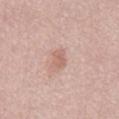biopsy_status: not biopsied; imaged during a skin examination
site: mid back
lesion_size:
  long_diameter_mm_approx: 2.5
automated_metrics:
  vs_skin_darker_L: 9.0
lighting: white-light
image:
  source: total-body photography crop
  field_of_view_mm: 15
patient:
  sex: female
  age_approx: 65